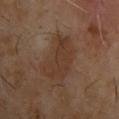Recorded during total-body skin imaging; not selected for excision or biopsy.
Longest diameter approximately 6 mm.
A lesion tile, about 15 mm wide, cut from a 3D total-body photograph.
A male patient in their mid-60s.
Automated tile analysis of the lesion measured an average lesion color of about L≈33 a*≈16 b*≈25 (CIELAB) and a lesion–skin lightness drop of about 6.
The tile uses cross-polarized illumination.
On the upper back.